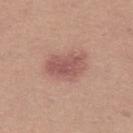The lesion was tiled from a total-body skin photograph and was not biopsied.
The lesion is located on the left thigh.
The patient is a female aged approximately 20.
A lesion tile, about 15 mm wide, cut from a 3D total-body photograph.
The lesion-visualizer software estimated a mean CIELAB color near L≈54 a*≈23 b*≈24, a lesion–skin lightness drop of about 10, and a normalized border contrast of about 7. And it measured a classifier nevus-likeness of about 80/100 and a lesion-detection confidence of about 100/100.